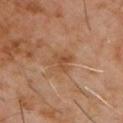follow-up — total-body-photography surveillance lesion; no biopsy
acquisition — ~15 mm tile from a whole-body skin photo
diameter — ≈3 mm
subject — male, aged around 60
tile lighting — cross-polarized
site — the chest